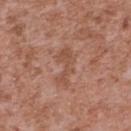automated lesion analysis: a mean CIELAB color near L≈51 a*≈22 b*≈29, roughly 7 lightness units darker than nearby skin, and a normalized border contrast of about 5.5
lesion diameter: ≈4.5 mm
illumination: white-light illumination
body site: the upper back
subject: male, in their mid-40s
image: total-body-photography crop, ~15 mm field of view